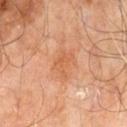follow-up: catalogued during a skin exam; not biopsied | image-analysis metrics: a lesion area of about 5.5 mm², an outline eccentricity of about 0.7 (0 = round, 1 = elongated), and a shape-asymmetry score of about 0.45 (0 = symmetric); a lesion–skin lightness drop of about 7 | subject: male, aged around 60 | illumination: cross-polarized | location: the leg | image: 15 mm crop, total-body photography | lesion size: about 3 mm.